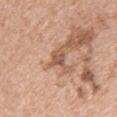Image and clinical context:
A 15 mm close-up extracted from a 3D total-body photography capture. A female subject, aged 38–42. The lesion-visualizer software estimated a lesion area of about 3.5 mm², a shape eccentricity near 0.8, and a symmetry-axis asymmetry near 0.2. It also reported a lesion color around L≈55 a*≈21 b*≈30 in CIELAB and about 11 CIELAB-L* units darker than the surrounding skin. And it measured a classifier nevus-likeness of about 0/100 and a detector confidence of about 100 out of 100 that the crop contains a lesion. This is a white-light tile. The lesion is on the chest.